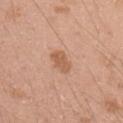The lesion was photographed on a routine skin check and not biopsied; there is no pathology result.
On the right upper arm.
The recorded lesion diameter is about 3 mm.
The subject is a male in their 20s.
This is a white-light tile.
A close-up tile cropped from a whole-body skin photograph, about 15 mm across.